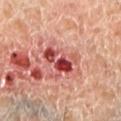biopsy status: catalogued during a skin exam; not biopsied
tile lighting: cross-polarized illumination
anatomic site: the left lower leg
diameter: ~5 mm (longest diameter)
patient: male, aged 53 to 57
image source: ~15 mm crop, total-body skin-cancer survey